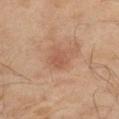workup = imaged on a skin check; not biopsied
imaging modality = ~15 mm crop, total-body skin-cancer survey
patient = male, aged 58–62
site = the leg
automated metrics = a footprint of about 4.5 mm² and an eccentricity of roughly 0.65
lesion size = about 3 mm